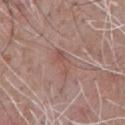biopsy_status: not biopsied; imaged during a skin examination
image:
  source: total-body photography crop
  field_of_view_mm: 15
site: front of the torso
lesion_size:
  long_diameter_mm_approx: 4.0
lighting: white-light
patient:
  sex: male
  age_approx: 70